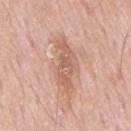{
  "biopsy_status": "not biopsied; imaged during a skin examination",
  "site": "mid back",
  "image": {
    "source": "total-body photography crop",
    "field_of_view_mm": 15
  },
  "lesion_size": {
    "long_diameter_mm_approx": 7.0
  },
  "patient": {
    "sex": "male",
    "age_approx": 65
  }
}